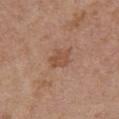Background:
The lesion is on the abdomen. A female subject in their mid- to late 60s. A roughly 15 mm field-of-view crop from a total-body skin photograph. Longest diameter approximately 2.5 mm. Imaged with white-light lighting.